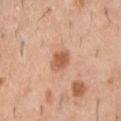<tbp_lesion>
<biopsy_status>not biopsied; imaged during a skin examination</biopsy_status>
<image>
  <source>total-body photography crop</source>
  <field_of_view_mm>15</field_of_view_mm>
</image>
<patient>
  <sex>male</sex>
  <age_approx>60</age_approx>
</patient>
<site>back</site>
<automated_metrics>
  <border_irregularity_0_10>1.5</border_irregularity_0_10>
  <color_variation_0_10>2.5</color_variation_0_10>
  <peripheral_color_asymmetry>1.0</peripheral_color_asymmetry>
</automated_metrics>
<lighting>white-light</lighting>
<lesion_size>
  <long_diameter_mm_approx>2.5</long_diameter_mm_approx>
</lesion_size>
</tbp_lesion>